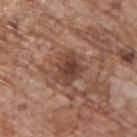The lesion was tiled from a total-body skin photograph and was not biopsied. A 15 mm close-up extracted from a 3D total-body photography capture. About 4 mm across. Located on the upper back. A male subject aged 68–72.